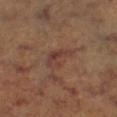{"biopsy_status": "not biopsied; imaged during a skin examination", "patient": {"sex": "female", "age_approx": 65}, "automated_metrics": {"cielab_L": 36, "cielab_a": 19, "cielab_b": 23, "vs_skin_darker_L": 6.0, "vs_skin_contrast_norm": 6.0, "border_irregularity_0_10": 3.0, "color_variation_0_10": 4.0, "peripheral_color_asymmetry": 1.5, "lesion_detection_confidence_0_100": 80}, "image": {"source": "total-body photography crop", "field_of_view_mm": 15}, "lesion_size": {"long_diameter_mm_approx": 4.0}, "lighting": "cross-polarized", "site": "right lower leg"}